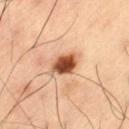follow-up: imaged on a skin check; not biopsied
imaging modality: ~15 mm crop, total-body skin-cancer survey
size: ~3.5 mm (longest diameter)
image-analysis metrics: a footprint of about 6.5 mm², a shape eccentricity near 0.65, and two-axis asymmetry of about 0.2; a mean CIELAB color near L≈51 a*≈26 b*≈37, about 22 CIELAB-L* units darker than the surrounding skin, and a normalized border contrast of about 14
patient: male, approximately 60 years of age
location: the left thigh
lighting: cross-polarized illumination